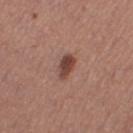Clinical impression: This lesion was catalogued during total-body skin photography and was not selected for biopsy. Clinical summary: This image is a 15 mm lesion crop taken from a total-body photograph. This is a white-light tile. The lesion's longest dimension is about 3 mm. A female subject, in their mid-30s. On the right thigh.